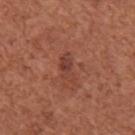• biopsy status — catalogued during a skin exam; not biopsied
• TBP lesion metrics — peripheral color asymmetry of about 0.5; an automated nevus-likeness rating near 0 out of 100 and a lesion-detection confidence of about 100/100
• tile lighting — white-light illumination
• size — ~3.5 mm (longest diameter)
• acquisition — total-body-photography crop, ~15 mm field of view
• body site — the right upper arm
• subject — female, aged around 50